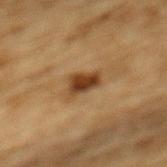Part of a total-body skin-imaging series; this lesion was reviewed on a skin check and was not flagged for biopsy. On the mid back. Approximately 3 mm at its widest. A 15 mm close-up extracted from a 3D total-body photography capture. A male subject aged 83 or older.